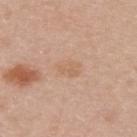notes — imaged on a skin check; not biopsied | patient — male, in their mid- to late 40s | tile lighting — white-light | size — about 3 mm | body site — the upper back | imaging modality — total-body-photography crop, ~15 mm field of view | TBP lesion metrics — an area of roughly 5 mm² and an eccentricity of roughly 0.75; an average lesion color of about L≈63 a*≈19 b*≈32 (CIELAB) and a lesion–skin lightness drop of about 5; a border-irregularity index near 3/10 and a peripheral color-asymmetry measure near 0.5.